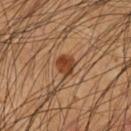Imaged during a routine full-body skin examination; the lesion was not biopsied and no histopathology is available.
Imaged with cross-polarized lighting.
The recorded lesion diameter is about 2.5 mm.
The lesion is on the front of the torso.
A male subject, approximately 55 years of age.
A 15 mm close-up tile from a total-body photography series done for melanoma screening.